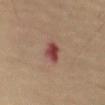  biopsy_status: not biopsied; imaged during a skin examination
  lesion_size:
    long_diameter_mm_approx: 3.0
  site: right upper arm
  patient:
    sex: male
    age_approx: 70
  image:
    source: total-body photography crop
    field_of_view_mm: 15
  automated_metrics:
    area_mm2_approx: 5.0
    eccentricity: 0.8
    cielab_L: 42
    cielab_a: 26
    cielab_b: 24
    vs_skin_darker_L: 13.0
    vs_skin_contrast_norm: 10.0
    nevus_likeness_0_100: 0
  lighting: cross-polarized